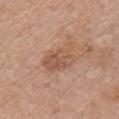Clinical impression: Recorded during total-body skin imaging; not selected for excision or biopsy. Image and clinical context: A close-up tile cropped from a whole-body skin photograph, about 15 mm across. Located on the chest. The patient is a male in their mid- to late 60s.